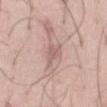Background: Captured under white-light illumination. Located on the front of the torso. A male patient, roughly 45 years of age. A 15 mm close-up extracted from a 3D total-body photography capture.